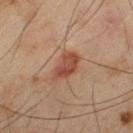Clinical impression:
The lesion was photographed on a routine skin check and not biopsied; there is no pathology result.
Clinical summary:
The recorded lesion diameter is about 4 mm. The tile uses cross-polarized illumination. A 15 mm close-up tile from a total-body photography series done for melanoma screening. A male subject about 45 years old. Located on the left thigh.